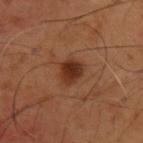biopsy status = total-body-photography surveillance lesion; no biopsy
location = the back
patient = male, in their mid-50s
acquisition = 15 mm crop, total-body photography